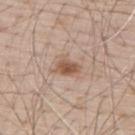The lesion was photographed on a routine skin check and not biopsied; there is no pathology result.
This image is a 15 mm lesion crop taken from a total-body photograph.
Measured at roughly 3 mm in maximum diameter.
A male patient about 70 years old.
The lesion is on the upper back.
The lesion-visualizer software estimated a lesion area of about 6 mm² and a symmetry-axis asymmetry near 0.35. The analysis additionally found a border-irregularity rating of about 3.5/10 and a color-variation rating of about 4/10. And it measured an automated nevus-likeness rating near 90 out of 100 and a detector confidence of about 100 out of 100 that the crop contains a lesion.